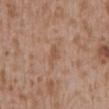| key | value |
|---|---|
| notes | no biopsy performed (imaged during a skin exam) |
| imaging modality | 15 mm crop, total-body photography |
| size | ≈3 mm |
| illumination | white-light illumination |
| patient | male, aged 43–47 |
| location | the abdomen |